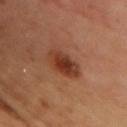<tbp_lesion>
<biopsy_status>not biopsied; imaged during a skin examination</biopsy_status>
<image>
  <source>total-body photography crop</source>
  <field_of_view_mm>15</field_of_view_mm>
</image>
<lighting>cross-polarized</lighting>
<patient>
  <sex>female</sex>
  <age_approx>60</age_approx>
</patient>
<lesion_size>
  <long_diameter_mm_approx>4.5</long_diameter_mm_approx>
</lesion_size>
<site>chest</site>
</tbp_lesion>